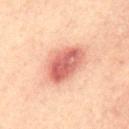<record>
  <biopsy_status>not biopsied; imaged during a skin examination</biopsy_status>
  <image>
    <source>total-body photography crop</source>
    <field_of_view_mm>15</field_of_view_mm>
  </image>
  <patient>
    <sex>male</sex>
    <age_approx>35</age_approx>
  </patient>
  <site>mid back</site>
  <lighting>cross-polarized</lighting>
</record>